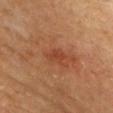Imaged during a routine full-body skin examination; the lesion was not biopsied and no histopathology is available.